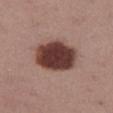The lesion was photographed on a routine skin check and not biopsied; there is no pathology result.
From the left thigh.
The subject is a female about 55 years old.
A 15 mm close-up extracted from a 3D total-body photography capture.
This is a white-light tile.